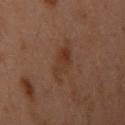Clinical impression:
This lesion was catalogued during total-body skin photography and was not selected for biopsy.
Clinical summary:
Cropped from a whole-body photographic skin survey; the tile spans about 15 mm. The lesion is located on the left arm. Measured at roughly 4 mm in maximum diameter. Automated image analysis of the tile measured a footprint of about 7 mm², a shape eccentricity near 0.85, and a symmetry-axis asymmetry near 0.3. And it measured an average lesion color of about L≈28 a*≈16 b*≈24 (CIELAB), roughly 5 lightness units darker than nearby skin, and a normalized border contrast of about 6. And it measured a border-irregularity rating of about 3/10, internal color variation of about 3.5 on a 0–10 scale, and radial color variation of about 1. And it measured a nevus-likeness score of about 45/100. A female subject in their mid- to late 50s.